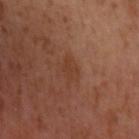Case summary:
– workup: imaged on a skin check; not biopsied
– image: ~15 mm tile from a whole-body skin photo
– diameter: about 2.5 mm
– site: the left upper arm
– automated lesion analysis: a footprint of about 4.5 mm², a shape eccentricity near 0.65, and a shape-asymmetry score of about 0.3 (0 = symmetric); an average lesion color of about L≈38 a*≈22 b*≈30 (CIELAB) and a normalized border contrast of about 5; a border-irregularity rating of about 3.5/10, a within-lesion color-variation index near 1.5/10, and peripheral color asymmetry of about 0.5; a nevus-likeness score of about 0/100 and a lesion-detection confidence of about 100/100
– patient: female, about 40 years old